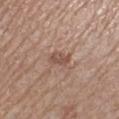Case summary:
* workup · no biopsy performed (imaged during a skin exam)
* automated lesion analysis · a footprint of about 4 mm², an outline eccentricity of about 0.7 (0 = round, 1 = elongated), and a shape-asymmetry score of about 0.25 (0 = symmetric)
* acquisition · 15 mm crop, total-body photography
* subject · female, approximately 55 years of age
* tile lighting · white-light illumination
* site · the right lower leg
* lesion size · about 2.5 mm